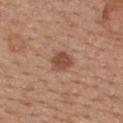Impression:
This lesion was catalogued during total-body skin photography and was not selected for biopsy.
Background:
A region of skin cropped from a whole-body photographic capture, roughly 15 mm wide. A female patient, about 35 years old. From the upper back. Measured at roughly 3 mm in maximum diameter.